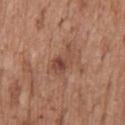Assessment:
The lesion was tiled from a total-body skin photograph and was not biopsied.
Acquisition and patient details:
Imaged with white-light lighting. The lesion is on the mid back. Approximately 4 mm at its widest. Cropped from a total-body skin-imaging series; the visible field is about 15 mm. A male subject roughly 60 years of age. An algorithmic analysis of the crop reported a footprint of about 6 mm². The software also gave a border-irregularity rating of about 4.5/10, internal color variation of about 5 on a 0–10 scale, and a peripheral color-asymmetry measure near 1.5. The analysis additionally found an automated nevus-likeness rating near 10 out of 100.